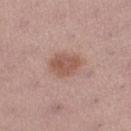Captured during whole-body skin photography for melanoma surveillance; the lesion was not biopsied.
From the left lower leg.
This is a white-light tile.
The subject is a female aged around 55.
The lesion-visualizer software estimated a classifier nevus-likeness of about 75/100.
A 15 mm close-up extracted from a 3D total-body photography capture.
Longest diameter approximately 4 mm.